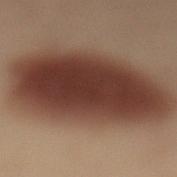<tbp_lesion>
  <biopsy_status>not biopsied; imaged during a skin examination</biopsy_status>
  <patient>
    <sex>male</sex>
    <age_approx>55</age_approx>
  </patient>
  <site>left lower leg</site>
  <lighting>cross-polarized</lighting>
  <image>
    <source>total-body photography crop</source>
    <field_of_view_mm>15</field_of_view_mm>
  </image>
</tbp_lesion>